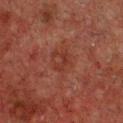Captured during whole-body skin photography for melanoma surveillance; the lesion was not biopsied. About 4.5 mm across. On the chest. A 15 mm close-up tile from a total-body photography series done for melanoma screening. The subject is a male aged 48–52. This is a cross-polarized tile. The total-body-photography lesion software estimated a lesion area of about 9 mm². It also reported internal color variation of about 3.5 on a 0–10 scale and a peripheral color-asymmetry measure near 1.